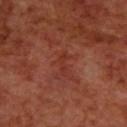Recorded during total-body skin imaging; not selected for excision or biopsy. Located on the upper back. A male patient, roughly 70 years of age. Cropped from a total-body skin-imaging series; the visible field is about 15 mm. The recorded lesion diameter is about 3 mm.